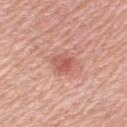{"biopsy_status": "not biopsied; imaged during a skin examination", "site": "left upper arm", "lighting": "white-light", "patient": {"sex": "female", "age_approx": 55}, "image": {"source": "total-body photography crop", "field_of_view_mm": 15}, "automated_metrics": {"area_mm2_approx": 5.5, "eccentricity": 0.75, "shape_asymmetry": 0.25, "border_irregularity_0_10": 2.5, "peripheral_color_asymmetry": 1.0, "lesion_detection_confidence_0_100": 100}}